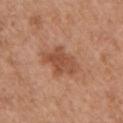Assessment:
Part of a total-body skin-imaging series; this lesion was reviewed on a skin check and was not flagged for biopsy.
Context:
A close-up tile cropped from a whole-body skin photograph, about 15 mm across. The tile uses white-light illumination. On the left upper arm. The total-body-photography lesion software estimated an area of roughly 11 mm², an outline eccentricity of about 0.8 (0 = round, 1 = elongated), and a shape-asymmetry score of about 0.3 (0 = symmetric). And it measured a lesion–skin lightness drop of about 10 and a normalized lesion–skin contrast near 7. A female subject in their mid-70s. The recorded lesion diameter is about 4.5 mm.